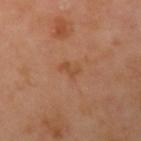workup: catalogued during a skin exam; not biopsied | image-analysis metrics: an area of roughly 3 mm², an outline eccentricity of about 0.8 (0 = round, 1 = elongated), and a symmetry-axis asymmetry near 0.5; a lesion color around L≈50 a*≈23 b*≈35 in CIELAB; a border-irregularity index near 5.5/10, internal color variation of about 0 on a 0–10 scale, and radial color variation of about 0; a nevus-likeness score of about 0/100 | lesion diameter: about 2.5 mm | lighting: cross-polarized | site: the left upper arm | image: ~15 mm crop, total-body skin-cancer survey | subject: female, in their mid-50s.